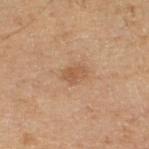Clinical impression: Captured during whole-body skin photography for melanoma surveillance; the lesion was not biopsied. Context: A 15 mm close-up extracted from a 3D total-body photography capture. The patient is a male roughly 70 years of age. The tile uses cross-polarized illumination. From the right lower leg. The lesion-visualizer software estimated a lesion area of about 3.5 mm², an outline eccentricity of about 0.8 (0 = round, 1 = elongated), and two-axis asymmetry of about 0.25. And it measured roughly 6 lightness units darker than nearby skin and a lesion-to-skin contrast of about 6 (normalized; higher = more distinct). And it measured a border-irregularity index near 2/10 and peripheral color asymmetry of about 0.5. The analysis additionally found a nevus-likeness score of about 25/100.